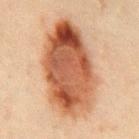| feature | finding |
|---|---|
| workup | catalogued during a skin exam; not biopsied |
| image source | 15 mm crop, total-body photography |
| patient | male, aged around 45 |
| body site | the abdomen |